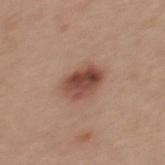Case summary:
• workup · total-body-photography surveillance lesion; no biopsy
• illumination · white-light illumination
• size · ≈4.5 mm
• image · ~15 mm tile from a whole-body skin photo
• patient · male, aged 28–32
• site · the mid back